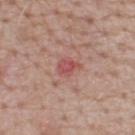site: upper back
image:
  source: total-body photography crop
  field_of_view_mm: 15
patient:
  sex: male
  age_approx: 65